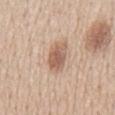  biopsy_status: not biopsied; imaged during a skin examination
  site: chest
  automated_metrics:
    cielab_L: 60
    cielab_a: 18
    cielab_b: 29
    vs_skin_darker_L: 12.0
    vs_skin_contrast_norm: 7.5
  patient:
    sex: male
    age_approx: 60
  image:
    source: total-body photography crop
    field_of_view_mm: 15
  lighting: white-light
  lesion_size:
    long_diameter_mm_approx: 4.5